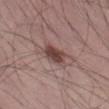Findings:
- notes — no biopsy performed (imaged during a skin exam)
- subject — male, in their mid- to late 30s
- lighting — white-light illumination
- acquisition — 15 mm crop, total-body photography
- lesion diameter — about 4 mm
- body site — the leg
- automated metrics — a lesion area of about 7 mm², a shape eccentricity near 0.8, and a symmetry-axis asymmetry near 0.25; roughly 12 lightness units darker than nearby skin and a normalized lesion–skin contrast near 9